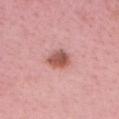The lesion was photographed on a routine skin check and not biopsied; there is no pathology result.
The recorded lesion diameter is about 3 mm.
The tile uses white-light illumination.
A lesion tile, about 15 mm wide, cut from a 3D total-body photograph.
The lesion is on the head or neck.
The lesion-visualizer software estimated an area of roughly 5.5 mm², an eccentricity of roughly 0.65, and a shape-asymmetry score of about 0.3 (0 = symmetric). The software also gave a lesion color around L≈55 a*≈27 b*≈26 in CIELAB and a normalized lesion–skin contrast near 9.5. The software also gave a border-irregularity index near 2.5/10, internal color variation of about 3.5 on a 0–10 scale, and a peripheral color-asymmetry measure near 1. The software also gave a lesion-detection confidence of about 100/100.
A female patient aged approximately 20.